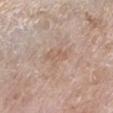The lesion was photographed on a routine skin check and not biopsied; there is no pathology result.